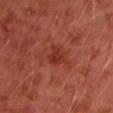Captured during whole-body skin photography for melanoma surveillance; the lesion was not biopsied. The lesion-visualizer software estimated an area of roughly 4.5 mm². The software also gave an average lesion color of about L≈34 a*≈31 b*≈31 (CIELAB) and a lesion-to-skin contrast of about 6.5 (normalized; higher = more distinct). The analysis additionally found a border-irregularity index near 3.5/10, a within-lesion color-variation index near 2/10, and peripheral color asymmetry of about 1. The lesion is located on the left upper arm. Approximately 2.5 mm at its widest. A male subject, aged 28–32. A 15 mm crop from a total-body photograph taken for skin-cancer surveillance. The tile uses cross-polarized illumination.